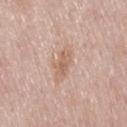This lesion was catalogued during total-body skin photography and was not selected for biopsy. The recorded lesion diameter is about 4 mm. The subject is a male aged 53–57. The lesion is located on the mid back. A 15 mm crop from a total-body photograph taken for skin-cancer surveillance. Imaged with white-light lighting.